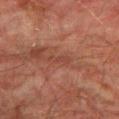Cropped from a whole-body photographic skin survey; the tile spans about 15 mm. The subject is a male aged around 75. From the left lower leg. Captured under cross-polarized illumination.